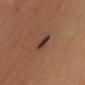Assessment:
The lesion was tiled from a total-body skin photograph and was not biopsied.
Background:
The tile uses cross-polarized illumination. A 15 mm close-up tile from a total-body photography series done for melanoma screening. The subject is a female roughly 40 years of age. About 2.5 mm across. Located on the head or neck. The lesion-visualizer software estimated an area of roughly 4.5 mm², an eccentricity of roughly 0.6, and two-axis asymmetry of about 0.25. The software also gave a border-irregularity index near 2.5/10 and internal color variation of about 6.5 on a 0–10 scale.